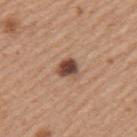Case summary:
• illumination — white-light illumination
• site — the left upper arm
• imaging modality — ~15 mm crop, total-body skin-cancer survey
• image-analysis metrics — an outline eccentricity of about 0.6 (0 = round, 1 = elongated) and a shape-asymmetry score of about 0.2 (0 = symmetric); border irregularity of about 2 on a 0–10 scale, a within-lesion color-variation index near 5/10, and radial color variation of about 1.5
• patient — male, roughly 55 years of age